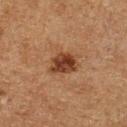| feature | finding |
|---|---|
| tile lighting | cross-polarized illumination |
| site | the left lower leg |
| acquisition | total-body-photography crop, ~15 mm field of view |
| lesion diameter | ≈3.5 mm |
| subject | male, aged 73–77 |
| automated lesion analysis | a mean CIELAB color near L≈32 a*≈19 b*≈27, a lesion–skin lightness drop of about 11, and a normalized lesion–skin contrast near 10.5 |